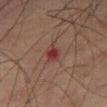Clinical impression: Recorded during total-body skin imaging; not selected for excision or biopsy. Background: The lesion's longest dimension is about 3 mm. The subject is a male in their 60s. Cropped from a total-body skin-imaging series; the visible field is about 15 mm. The tile uses cross-polarized illumination.